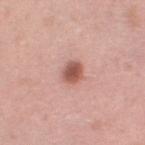Assessment: Recorded during total-body skin imaging; not selected for excision or biopsy. Image and clinical context: The lesion-visualizer software estimated a lesion–skin lightness drop of about 14 and a normalized lesion–skin contrast near 9. Captured under white-light illumination. About 3 mm across. A close-up tile cropped from a whole-body skin photograph, about 15 mm across. On the left thigh. A female subject, aged 38–42.